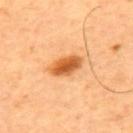Notes:
– TBP lesion metrics: a border-irregularity index near 1.5/10, a color-variation rating of about 4.5/10, and radial color variation of about 1.5; a nevus-likeness score of about 100/100
– image: total-body-photography crop, ~15 mm field of view
– size: ~4 mm (longest diameter)
– lighting: cross-polarized
– site: the upper back
– patient: male, about 65 years old